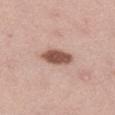This lesion was catalogued during total-body skin photography and was not selected for biopsy.
From the left thigh.
A female patient, in their mid-30s.
A roughly 15 mm field-of-view crop from a total-body skin photograph.